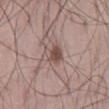Q: Was a biopsy performed?
A: catalogued during a skin exam; not biopsied
Q: What did automated image analysis measure?
A: a lesion color around L≈50 a*≈18 b*≈22 in CIELAB and a lesion-to-skin contrast of about 8 (normalized; higher = more distinct); border irregularity of about 3 on a 0–10 scale, a within-lesion color-variation index near 3.5/10, and a peripheral color-asymmetry measure near 1.5
Q: How large is the lesion?
A: about 3 mm
Q: What is the anatomic site?
A: the back
Q: How was this image acquired?
A: total-body-photography crop, ~15 mm field of view
Q: How was the tile lit?
A: white-light illumination
Q: Who is the patient?
A: male, about 45 years old